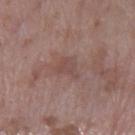| key | value |
|---|---|
| workup | total-body-photography surveillance lesion; no biopsy |
| imaging modality | total-body-photography crop, ~15 mm field of view |
| TBP lesion metrics | a lesion area of about 4.5 mm², an outline eccentricity of about 0.7 (0 = round, 1 = elongated), and a shape-asymmetry score of about 0.4 (0 = symmetric); a lesion color around L≈47 a*≈19 b*≈22 in CIELAB, roughly 6 lightness units darker than nearby skin, and a lesion-to-skin contrast of about 5 (normalized; higher = more distinct); a color-variation rating of about 1/10 and peripheral color asymmetry of about 0.5 |
| tile lighting | white-light illumination |
| subject | female, aged around 70 |
| size | about 3 mm |
| body site | the left lower leg |